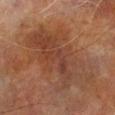No biopsy was performed on this lesion — it was imaged during a full skin examination and was not determined to be concerning. A male subject, aged approximately 70. Cropped from a total-body skin-imaging series; the visible field is about 15 mm. The lesion-visualizer software estimated a border-irregularity rating of about 8/10, a color-variation rating of about 4.5/10, and radial color variation of about 1.5. This is a cross-polarized tile. On the right lower leg. About 11 mm across.